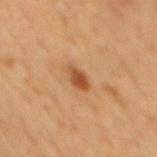The lesion was tiled from a total-body skin photograph and was not biopsied.
Imaged with cross-polarized lighting.
The lesion's longest dimension is about 3 mm.
The lesion is on the back.
A male patient, aged approximately 60.
A 15 mm close-up extracted from a 3D total-body photography capture.